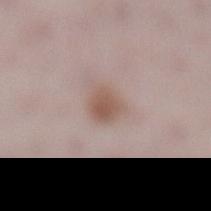{
  "biopsy_status": "not biopsied; imaged during a skin examination"
}